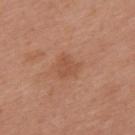{
  "biopsy_status": "not biopsied; imaged during a skin examination",
  "lighting": "white-light",
  "patient": {
    "sex": "female",
    "age_approx": 40
  },
  "automated_metrics": {
    "area_mm2_approx": 6.5,
    "eccentricity": 0.3,
    "cielab_L": 52,
    "cielab_a": 23,
    "cielab_b": 33,
    "vs_skin_darker_L": 6.0
  },
  "site": "upper back",
  "image": {
    "source": "total-body photography crop",
    "field_of_view_mm": 15
  }
}